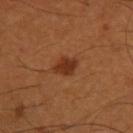Impression: Captured during whole-body skin photography for melanoma surveillance; the lesion was not biopsied. Context: The lesion is on the right thigh. Longest diameter approximately 3 mm. This is a cross-polarized tile. A male subject, about 50 years old. Cropped from a whole-body photographic skin survey; the tile spans about 15 mm.